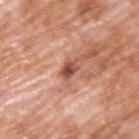The lesion was tiled from a total-body skin photograph and was not biopsied. This is a white-light tile. On the upper back. Cropped from a total-body skin-imaging series; the visible field is about 15 mm. A male patient, roughly 60 years of age. The lesion's longest dimension is about 2.5 mm.